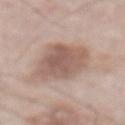{
  "biopsy_status": "not biopsied; imaged during a skin examination",
  "patient": {
    "sex": "male",
    "age_approx": 75
  },
  "image": {
    "source": "total-body photography crop",
    "field_of_view_mm": 15
  },
  "automated_metrics": {
    "cielab_L": 57,
    "cielab_a": 17,
    "cielab_b": 25,
    "vs_skin_darker_L": 11.0,
    "vs_skin_contrast_norm": 7.5
  },
  "lighting": "white-light",
  "site": "abdomen",
  "lesion_size": {
    "long_diameter_mm_approx": 6.5
  }
}